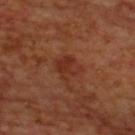Assessment: The lesion was photographed on a routine skin check and not biopsied; there is no pathology result. Context: Longest diameter approximately 3 mm. Located on the upper back. A close-up tile cropped from a whole-body skin photograph, about 15 mm across. The tile uses cross-polarized illumination. The subject is a male approximately 70 years of age.